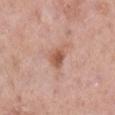Q: Is there a histopathology result?
A: no biopsy performed (imaged during a skin exam)
Q: What is the anatomic site?
A: the right lower leg
Q: What did automated image analysis measure?
A: a lesion area of about 5 mm² and an eccentricity of roughly 0.65; a nevus-likeness score of about 20/100 and a detector confidence of about 100 out of 100 that the crop contains a lesion
Q: What kind of image is this?
A: 15 mm crop, total-body photography
Q: Patient demographics?
A: female, about 50 years old
Q: Illumination type?
A: white-light illumination
Q: Lesion size?
A: ≈3 mm